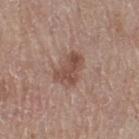<record>
  <biopsy_status>not biopsied; imaged during a skin examination</biopsy_status>
  <lighting>white-light</lighting>
  <lesion_size>
    <long_diameter_mm_approx>4.0</long_diameter_mm_approx>
  </lesion_size>
  <automated_metrics>
    <cielab_L>49</cielab_L>
    <cielab_a>19</cielab_a>
    <cielab_b>25</cielab_b>
    <vs_skin_contrast_norm>7.0</vs_skin_contrast_norm>
    <nevus_likeness_0_100>25</nevus_likeness_0_100>
    <lesion_detection_confidence_0_100>100</lesion_detection_confidence_0_100>
  </automated_metrics>
  <site>right thigh</site>
  <image>
    <source>total-body photography crop</source>
    <field_of_view_mm>15</field_of_view_mm>
  </image>
  <patient>
    <sex>male</sex>
    <age_approx>65</age_approx>
  </patient>
</record>